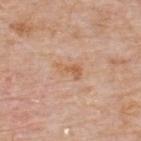The lesion was tiled from a total-body skin photograph and was not biopsied.
On the upper back.
A male patient, aged 73 to 77.
Captured under white-light illumination.
A roughly 15 mm field-of-view crop from a total-body skin photograph.
Longest diameter approximately 3 mm.